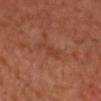Imaged during a routine full-body skin examination; the lesion was not biopsied and no histopathology is available. Imaged with cross-polarized lighting. Located on the chest. The total-body-photography lesion software estimated a classifier nevus-likeness of about 0/100 and a detector confidence of about 95 out of 100 that the crop contains a lesion. Measured at roughly 3.5 mm in maximum diameter. A male patient, aged approximately 60. A 15 mm crop from a total-body photograph taken for skin-cancer surveillance.